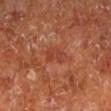Q: Was this lesion biopsied?
A: catalogued during a skin exam; not biopsied
Q: Where on the body is the lesion?
A: the leg
Q: Patient demographics?
A: male, in their mid- to late 60s
Q: What is the imaging modality?
A: 15 mm crop, total-body photography